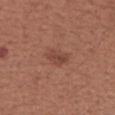Clinical impression:
This lesion was catalogued during total-body skin photography and was not selected for biopsy.